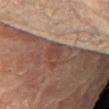  biopsy_status: not biopsied; imaged during a skin examination
  patient:
    sex: female
    age_approx: 80
  site: right arm
  image:
    source: total-body photography crop
    field_of_view_mm: 15